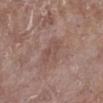Q: Was a biopsy performed?
A: imaged on a skin check; not biopsied
Q: What is the lesion's diameter?
A: about 3.5 mm
Q: Who is the patient?
A: female, aged around 75
Q: What kind of image is this?
A: ~15 mm tile from a whole-body skin photo
Q: Automated lesion metrics?
A: a footprint of about 6.5 mm² and an outline eccentricity of about 0.75 (0 = round, 1 = elongated); an average lesion color of about L≈50 a*≈19 b*≈23 (CIELAB), a lesion–skin lightness drop of about 6, and a normalized lesion–skin contrast near 4.5
Q: Where on the body is the lesion?
A: the right lower leg
Q: Illumination type?
A: white-light